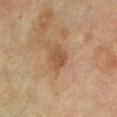Part of a total-body skin-imaging series; this lesion was reviewed on a skin check and was not flagged for biopsy.
A female subject, in their 70s.
A roughly 15 mm field-of-view crop from a total-body skin photograph.
Measured at roughly 2.5 mm in maximum diameter.
Located on the left lower leg.
An algorithmic analysis of the crop reported an area of roughly 4.5 mm², a shape eccentricity near 0.6, and a shape-asymmetry score of about 0.3 (0 = symmetric). The analysis additionally found a border-irregularity rating of about 2.5/10, a within-lesion color-variation index near 2/10, and peripheral color asymmetry of about 0.5. The analysis additionally found a nevus-likeness score of about 20/100 and lesion-presence confidence of about 100/100.
This is a cross-polarized tile.